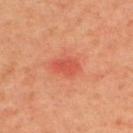- follow-up · total-body-photography surveillance lesion; no biopsy
- image · total-body-photography crop, ~15 mm field of view
- site · the upper back
- automated metrics · a border-irregularity index near 2/10, a within-lesion color-variation index near 1.5/10, and peripheral color asymmetry of about 0.5
- size · ~3 mm (longest diameter)
- tile lighting · cross-polarized
- subject · male, approximately 65 years of age